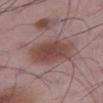Assessment: No biopsy was performed on this lesion — it was imaged during a full skin examination and was not determined to be concerning. Background: Captured under white-light illumination. The lesion is on the right thigh. Automated image analysis of the tile measured a border-irregularity rating of about 2.5/10 and radial color variation of about 1. About 5.5 mm across. A male patient aged around 50. A close-up tile cropped from a whole-body skin photograph, about 15 mm across.